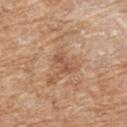Q: Was this lesion biopsied?
A: no biopsy performed (imaged during a skin exam)
Q: How was this image acquired?
A: total-body-photography crop, ~15 mm field of view
Q: Illumination type?
A: white-light illumination
Q: Who is the patient?
A: female, aged 68–72
Q: How large is the lesion?
A: ≈3 mm
Q: Where on the body is the lesion?
A: the upper back